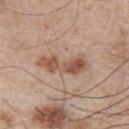follow-up: imaged on a skin check; not biopsied
patient: male, about 65 years old
illumination: white-light illumination
size: ≈5.5 mm
site: the right upper arm
automated lesion analysis: a footprint of about 9 mm², a shape eccentricity near 0.95, and a symmetry-axis asymmetry near 0.35; a within-lesion color-variation index near 4/10 and a peripheral color-asymmetry measure near 1; a nevus-likeness score of about 60/100 and lesion-presence confidence of about 100/100
acquisition: ~15 mm crop, total-body skin-cancer survey